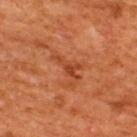Assessment:
Imaged during a routine full-body skin examination; the lesion was not biopsied and no histopathology is available.
Background:
A region of skin cropped from a whole-body photographic capture, roughly 15 mm wide. A male subject, aged around 65. The lesion-visualizer software estimated border irregularity of about 8.5 on a 0–10 scale and peripheral color asymmetry of about 0.5. Captured under cross-polarized illumination. From the upper back.